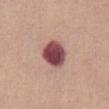Clinical impression: This lesion was catalogued during total-body skin photography and was not selected for biopsy. Context: Imaged with white-light lighting. Located on the front of the torso. The subject is a female aged approximately 65. The lesion's longest dimension is about 4 mm. A close-up tile cropped from a whole-body skin photograph, about 15 mm across.